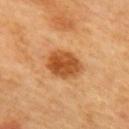{"image": {"source": "total-body photography crop", "field_of_view_mm": 15}, "lesion_size": {"long_diameter_mm_approx": 4.0}, "automated_metrics": {"area_mm2_approx": 11.0, "shape_asymmetry": 0.15, "cielab_L": 54, "cielab_a": 28, "cielab_b": 45, "vs_skin_darker_L": 15.0, "border_irregularity_0_10": 1.5, "color_variation_0_10": 4.5, "peripheral_color_asymmetry": 1.5, "nevus_likeness_0_100": 100, "lesion_detection_confidence_0_100": 100}, "lighting": "cross-polarized", "patient": {"sex": "female", "age_approx": 60}, "site": "upper back"}